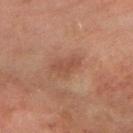| key | value |
|---|---|
| notes | total-body-photography surveillance lesion; no biopsy |
| lesion size | ~3.5 mm (longest diameter) |
| imaging modality | ~15 mm tile from a whole-body skin photo |
| automated metrics | a border-irregularity rating of about 3/10, a within-lesion color-variation index near 2/10, and peripheral color asymmetry of about 0.5 |
| subject | female, about 60 years old |
| lighting | cross-polarized |
| location | the arm |